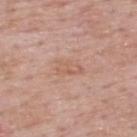This lesion was catalogued during total-body skin photography and was not selected for biopsy. From the upper back. An algorithmic analysis of the crop reported a lesion area of about 5 mm² and two-axis asymmetry of about 0.35. The analysis additionally found a lesion color around L≈60 a*≈21 b*≈29 in CIELAB, roughly 6 lightness units darker than nearby skin, and a normalized border contrast of about 4.5. The analysis additionally found border irregularity of about 3.5 on a 0–10 scale, internal color variation of about 2 on a 0–10 scale, and peripheral color asymmetry of about 1. And it measured a classifier nevus-likeness of about 0/100 and a detector confidence of about 100 out of 100 that the crop contains a lesion. This is a white-light tile. A 15 mm close-up tile from a total-body photography series done for melanoma screening. The patient is a male in their mid-50s. About 3.5 mm across.